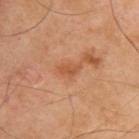Impression:
Part of a total-body skin-imaging series; this lesion was reviewed on a skin check and was not flagged for biopsy.
Context:
The tile uses cross-polarized illumination. A male patient, aged around 40. Cropped from a whole-body photographic skin survey; the tile spans about 15 mm. Automated tile analysis of the lesion measured a lesion area of about 3 mm², an eccentricity of roughly 0.85, and a symmetry-axis asymmetry near 0.4. The analysis additionally found about 8 CIELAB-L* units darker than the surrounding skin and a normalized lesion–skin contrast near 6. It also reported an automated nevus-likeness rating near 0 out of 100 and a lesion-detection confidence of about 100/100. The lesion's longest dimension is about 2.5 mm. From the upper back.